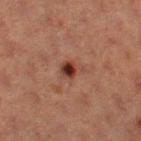Imaged during a routine full-body skin examination; the lesion was not biopsied and no histopathology is available. A lesion tile, about 15 mm wide, cut from a 3D total-body photograph. On the left thigh. A female patient aged 38–42.